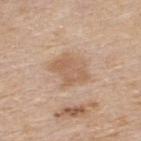Notes:
• workup — total-body-photography surveillance lesion; no biopsy
• image source — 15 mm crop, total-body photography
• illumination — white-light illumination
• patient — male, about 80 years old
• automated metrics — a lesion color around L≈60 a*≈18 b*≈32 in CIELAB, a lesion–skin lightness drop of about 8, and a normalized border contrast of about 6
• anatomic site — the upper back